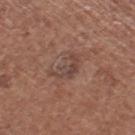{
  "biopsy_status": "not biopsied; imaged during a skin examination",
  "patient": {
    "sex": "female",
    "age_approx": 50
  },
  "lesion_size": {
    "long_diameter_mm_approx": 4.5
  },
  "lighting": "white-light",
  "site": "leg",
  "automated_metrics": {
    "eccentricity": 0.85,
    "vs_skin_contrast_norm": 5.5
  },
  "image": {
    "source": "total-body photography crop",
    "field_of_view_mm": 15
  }
}